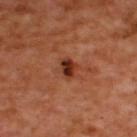  biopsy_status: not biopsied; imaged during a skin examination
  lighting: cross-polarized
  image:
    source: total-body photography crop
    field_of_view_mm: 15
  patient:
    sex: male
    age_approx: 50
  site: upper back